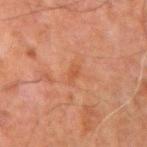Captured during whole-body skin photography for melanoma surveillance; the lesion was not biopsied. Located on the left arm. An algorithmic analysis of the crop reported an average lesion color of about L≈41 a*≈21 b*≈30 (CIELAB) and a lesion-to-skin contrast of about 5 (normalized; higher = more distinct). It also reported a detector confidence of about 100 out of 100 that the crop contains a lesion. The patient is a male approximately 60 years of age. The recorded lesion diameter is about 2.5 mm. Imaged with cross-polarized lighting. A lesion tile, about 15 mm wide, cut from a 3D total-body photograph.